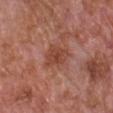Recorded during total-body skin imaging; not selected for excision or biopsy.
The tile uses white-light illumination.
On the chest.
Longest diameter approximately 3.5 mm.
A 15 mm crop from a total-body photograph taken for skin-cancer surveillance.
A male subject in their mid- to late 60s.